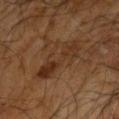biopsy status: total-body-photography surveillance lesion; no biopsy | patient: male, aged 58–62 | body site: the right arm | image source: total-body-photography crop, ~15 mm field of view | lesion size: ~6.5 mm (longest diameter) | automated metrics: an area of roughly 11 mm², an outline eccentricity of about 0.95 (0 = round, 1 = elongated), and a symmetry-axis asymmetry near 0.4; a border-irregularity rating of about 7/10 and a within-lesion color-variation index near 5.5/10.